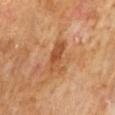A 15 mm close-up extracted from a 3D total-body photography capture. Captured under cross-polarized illumination. A male patient, roughly 60 years of age. The lesion's longest dimension is about 4.5 mm. From the abdomen.